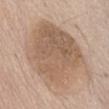The lesion's longest dimension is about 9 mm. A male patient roughly 80 years of age. Imaged with white-light lighting. A lesion tile, about 15 mm wide, cut from a 3D total-body photograph. The lesion is located on the abdomen.